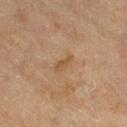This lesion was catalogued during total-body skin photography and was not selected for biopsy. Automated image analysis of the tile measured a shape eccentricity near 0.9 and a shape-asymmetry score of about 0.25 (0 = symmetric). The software also gave a classifier nevus-likeness of about 0/100. The lesion is on the right thigh. A female subject aged approximately 60. This is a cross-polarized tile. A close-up tile cropped from a whole-body skin photograph, about 15 mm across.